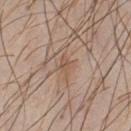  automated_metrics:
    area_mm2_approx: 3.0
    shape_asymmetry: 0.35
    cielab_L: 54
    cielab_a: 17
    cielab_b: 29
    vs_skin_darker_L: 7.0
    vs_skin_contrast_norm: 5.5
  site: chest
  image:
    source: total-body photography crop
    field_of_view_mm: 15
  patient:
    sex: male
    age_approx: 40
  lighting: white-light
  lesion_size:
    long_diameter_mm_approx: 2.5